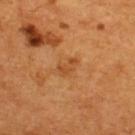| key | value |
|---|---|
| subject | male, about 55 years old |
| body site | the upper back |
| imaging modality | ~15 mm tile from a whole-body skin photo |
| TBP lesion metrics | an outline eccentricity of about 0.8 (0 = round, 1 = elongated); an average lesion color of about L≈45 a*≈24 b*≈39 (CIELAB), a lesion–skin lightness drop of about 7, and a normalized lesion–skin contrast near 5.5; a border-irregularity rating of about 3.5/10, internal color variation of about 1.5 on a 0–10 scale, and peripheral color asymmetry of about 0.5; an automated nevus-likeness rating near 0 out of 100 and a lesion-detection confidence of about 100/100 |
| tile lighting | cross-polarized illumination |
| lesion diameter | ~2.5 mm (longest diameter) |